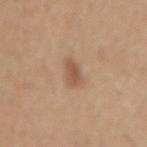{
  "biopsy_status": "not biopsied; imaged during a skin examination",
  "image": {
    "source": "total-body photography crop",
    "field_of_view_mm": 15
  },
  "site": "lower back",
  "patient": {
    "sex": "male",
    "age_approx": 40
  },
  "lesion_size": {
    "long_diameter_mm_approx": 2.5
  },
  "lighting": "white-light"
}